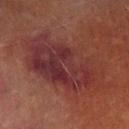Case summary:
• notes · catalogued during a skin exam; not biopsied
• site · the right lower leg
• patient · male, approximately 70 years of age
• image source · total-body-photography crop, ~15 mm field of view